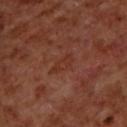Clinical impression: The lesion was photographed on a routine skin check and not biopsied; there is no pathology result. Context: A male patient, roughly 70 years of age. Captured under cross-polarized illumination. About 3 mm across. From the back. This image is a 15 mm lesion crop taken from a total-body photograph.